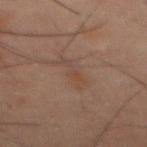Impression:
Recorded during total-body skin imaging; not selected for excision or biopsy.
Image and clinical context:
Cropped from a whole-body photographic skin survey; the tile spans about 15 mm. Automated image analysis of the tile measured an outline eccentricity of about 0.95 (0 = round, 1 = elongated) and a shape-asymmetry score of about 0.4 (0 = symmetric). The software also gave roughly 4 lightness units darker than nearby skin. The analysis additionally found a lesion-detection confidence of about 100/100. A male subject, approximately 60 years of age. From the front of the torso. About 4 mm across.